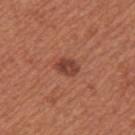Captured during whole-body skin photography for melanoma surveillance; the lesion was not biopsied.
Automated image analysis of the tile measured an eccentricity of roughly 0.75 and a shape-asymmetry score of about 0.2 (0 = symmetric). The analysis additionally found a lesion color around L≈43 a*≈26 b*≈29 in CIELAB, roughly 10 lightness units darker than nearby skin, and a normalized lesion–skin contrast near 8. The software also gave a classifier nevus-likeness of about 90/100 and a detector confidence of about 100 out of 100 that the crop contains a lesion.
The lesion is located on the left upper arm.
The tile uses white-light illumination.
A female subject, aged around 65.
A roughly 15 mm field-of-view crop from a total-body skin photograph.